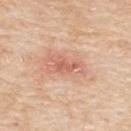Findings:
* illumination: white-light illumination
* subject: female, aged around 70
* lesion diameter: ~3 mm (longest diameter)
* anatomic site: the upper back
* image source: ~15 mm crop, total-body skin-cancer survey
* automated metrics: a lesion color around L≈62 a*≈26 b*≈31 in CIELAB, about 9 CIELAB-L* units darker than the surrounding skin, and a normalized lesion–skin contrast near 6; internal color variation of about 2.5 on a 0–10 scale and a peripheral color-asymmetry measure near 0.5; a classifier nevus-likeness of about 0/100 and lesion-presence confidence of about 100/100